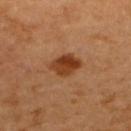Captured during whole-body skin photography for melanoma surveillance; the lesion was not biopsied.
On the back.
A male patient aged approximately 60.
Imaged with cross-polarized lighting.
Cropped from a whole-body photographic skin survey; the tile spans about 15 mm.